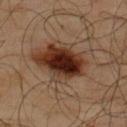<case>
  <biopsy_status>not biopsied; imaged during a skin examination</biopsy_status>
  <lighting>cross-polarized</lighting>
  <lesion_size>
    <long_diameter_mm_approx>4.0</long_diameter_mm_approx>
  </lesion_size>
  <patient>
    <sex>male</sex>
    <age_approx>65</age_approx>
  </patient>
  <image>
    <source>total-body photography crop</source>
    <field_of_view_mm>15</field_of_view_mm>
  </image>
  <site>back</site>
  <automated_metrics>
    <area_mm2_approx>8.0</area_mm2_approx>
    <shape_asymmetry>0.2</shape_asymmetry>
    <nevus_likeness_0_100>100</nevus_likeness_0_100>
    <lesion_detection_confidence_0_100>100</lesion_detection_confidence_0_100>
  </automated_metrics>
</case>